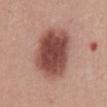Captured during whole-body skin photography for melanoma surveillance; the lesion was not biopsied.
On the abdomen.
Longest diameter approximately 7 mm.
Captured under white-light illumination.
This image is a 15 mm lesion crop taken from a total-body photograph.
A male patient, aged 53–57.